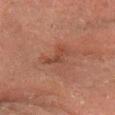Part of a total-body skin-imaging series; this lesion was reviewed on a skin check and was not flagged for biopsy. This is a cross-polarized tile. Measured at roughly 3 mm in maximum diameter. A male patient aged 68–72. The lesion is on the left forearm. A 15 mm crop from a total-body photograph taken for skin-cancer surveillance.